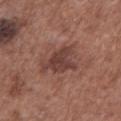{
  "patient": {
    "sex": "male",
    "age_approx": 75
  },
  "automated_metrics": {
    "area_mm2_approx": 12.0,
    "eccentricity": 0.75,
    "shape_asymmetry": 0.4,
    "color_variation_0_10": 3.0,
    "peripheral_color_asymmetry": 1.0
  },
  "lesion_size": {
    "long_diameter_mm_approx": 5.5
  },
  "lighting": "white-light",
  "site": "chest",
  "image": {
    "source": "total-body photography crop",
    "field_of_view_mm": 15
  }
}